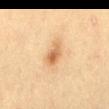notes: catalogued during a skin exam; not biopsied
patient: female, aged 53 to 57
site: the mid back
lesion diameter: ~3 mm (longest diameter)
imaging modality: total-body-photography crop, ~15 mm field of view